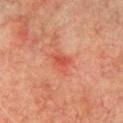– biopsy status · catalogued during a skin exam; not biopsied
– lesion diameter · ~3 mm (longest diameter)
– imaging modality · ~15 mm crop, total-body skin-cancer survey
– lighting · cross-polarized
– patient · male, in their mid-70s
– image-analysis metrics · an area of roughly 4.5 mm², an eccentricity of roughly 0.6, and a shape-asymmetry score of about 0.3 (0 = symmetric); about 7 CIELAB-L* units darker than the surrounding skin and a normalized lesion–skin contrast near 6; a border-irregularity index near 3/10, a color-variation rating of about 2/10, and peripheral color asymmetry of about 1
– anatomic site · the chest